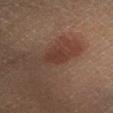Case summary:
- biopsy status · total-body-photography surveillance lesion; no biopsy
- automated lesion analysis · a nevus-likeness score of about 10/100 and a lesion-detection confidence of about 100/100
- image source · 15 mm crop, total-body photography
- site · the leg
- lesion size · ≈2.5 mm
- illumination · cross-polarized
- subject · male, in their 40s